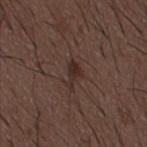<tbp_lesion>
  <biopsy_status>not biopsied; imaged during a skin examination</biopsy_status>
</tbp_lesion>